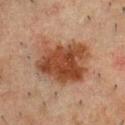Captured during whole-body skin photography for melanoma surveillance; the lesion was not biopsied.
The lesion is located on the chest.
This is a cross-polarized tile.
This image is a 15 mm lesion crop taken from a total-body photograph.
Longest diameter approximately 6.5 mm.
A male subject, about 50 years old.
Automated tile analysis of the lesion measured a border-irregularity rating of about 3/10 and peripheral color asymmetry of about 1.5. It also reported a lesion-detection confidence of about 100/100.